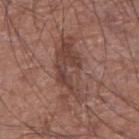Recorded during total-body skin imaging; not selected for excision or biopsy.
A male patient roughly 75 years of age.
Cropped from a whole-body photographic skin survey; the tile spans about 15 mm.
About 7.5 mm across.
The lesion is located on the arm.